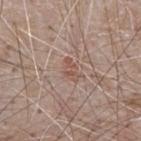A male subject, aged 63 to 67.
From the chest.
Captured under white-light illumination.
About 3 mm across.
Cropped from a whole-body photographic skin survey; the tile spans about 15 mm.